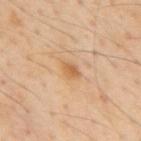subject: male, in their mid- to late 50s | image: ~15 mm crop, total-body skin-cancer survey | illumination: cross-polarized illumination | TBP lesion metrics: an area of roughly 3.5 mm², an outline eccentricity of about 0.75 (0 = round, 1 = elongated), and a symmetry-axis asymmetry near 0.3; a lesion color around L≈62 a*≈20 b*≈40 in CIELAB, about 8 CIELAB-L* units darker than the surrounding skin, and a normalized lesion–skin contrast near 6.5; internal color variation of about 2.5 on a 0–10 scale and a peripheral color-asymmetry measure near 0.5 | lesion size: ≈2.5 mm | body site: the mid back.